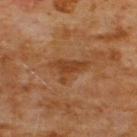* body site: the chest
* diameter: about 4.5 mm
* lighting: cross-polarized
* patient: male, about 60 years old
* image source: 15 mm crop, total-body photography